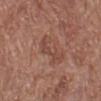notes=catalogued during a skin exam; not biopsied
tile lighting=white-light
TBP lesion metrics=a shape eccentricity near 0.9 and a symmetry-axis asymmetry near 0.5; a border-irregularity index near 7.5/10, internal color variation of about 2 on a 0–10 scale, and peripheral color asymmetry of about 0.5
subject=female, aged around 80
lesion size=about 4.5 mm
location=the left lower leg
image source=~15 mm crop, total-body skin-cancer survey